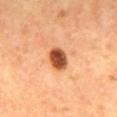biopsy status=imaged on a skin check; not biopsied | image source=~15 mm crop, total-body skin-cancer survey | anatomic site=the mid back | illumination=cross-polarized | subject=male, aged around 65 | TBP lesion metrics=an average lesion color of about L≈53 a*≈30 b*≈40 (CIELAB); a border-irregularity rating of about 1.5/10, internal color variation of about 5 on a 0–10 scale, and peripheral color asymmetry of about 1.5 | lesion size=~3 mm (longest diameter).